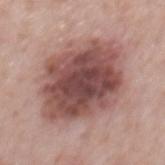Clinical impression:
The lesion was tiled from a total-body skin photograph and was not biopsied.
Clinical summary:
Located on the mid back. Imaged with white-light lighting. Automated tile analysis of the lesion measured a footprint of about 45 mm² and an eccentricity of roughly 0.6. A male subject, aged approximately 65. A 15 mm close-up extracted from a 3D total-body photography capture. Approximately 8.5 mm at its widest.